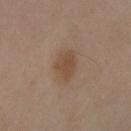Assessment:
Imaged during a routine full-body skin examination; the lesion was not biopsied and no histopathology is available.
Acquisition and patient details:
Captured under cross-polarized illumination. Located on the right upper arm. A female subject about 55 years old. The total-body-photography lesion software estimated a border-irregularity rating of about 2/10. A lesion tile, about 15 mm wide, cut from a 3D total-body photograph.